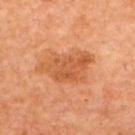follow-up: total-body-photography surveillance lesion; no biopsy | image: total-body-photography crop, ~15 mm field of view | tile lighting: cross-polarized | site: the upper back | patient: female, aged 63–67.